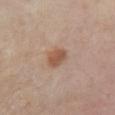Part of a total-body skin-imaging series; this lesion was reviewed on a skin check and was not flagged for biopsy.
About 3 mm across.
A female patient roughly 55 years of age.
On the leg.
A lesion tile, about 15 mm wide, cut from a 3D total-body photograph.